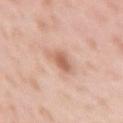Captured during whole-body skin photography for melanoma surveillance; the lesion was not biopsied. A female subject, aged 38–42. Longest diameter approximately 2.5 mm. The lesion is on the arm. A lesion tile, about 15 mm wide, cut from a 3D total-body photograph. The tile uses white-light illumination.